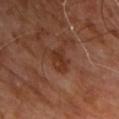follow-up = catalogued during a skin exam; not biopsied | body site = the upper back | illumination = cross-polarized | diameter = ~2.5 mm (longest diameter) | patient = male, approximately 60 years of age | image = ~15 mm crop, total-body skin-cancer survey.